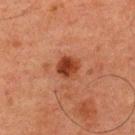Imaged during a routine full-body skin examination; the lesion was not biopsied and no histopathology is available. This image is a 15 mm lesion crop taken from a total-body photograph. A male patient in their 60s. The lesion is located on the back.